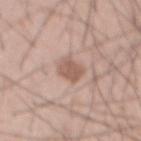follow-up: total-body-photography surveillance lesion; no biopsy
site: the abdomen
image: ~15 mm crop, total-body skin-cancer survey
tile lighting: white-light
size: ~2.5 mm (longest diameter)
patient: male, aged around 55